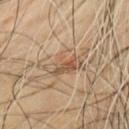The lesion was photographed on a routine skin check and not biopsied; there is no pathology result.
The recorded lesion diameter is about 5.5 mm.
The lesion is located on the chest.
Cropped from a total-body skin-imaging series; the visible field is about 15 mm.
A male patient aged 43–47.
This is a cross-polarized tile.